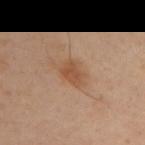{
  "biopsy_status": "not biopsied; imaged during a skin examination",
  "image": {
    "source": "total-body photography crop",
    "field_of_view_mm": 15
  },
  "patient": {
    "sex": "male",
    "age_approx": 45
  },
  "site": "arm"
}